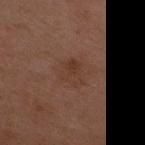Assessment:
Imaged during a routine full-body skin examination; the lesion was not biopsied and no histopathology is available.
Image and clinical context:
Approximately 3 mm at its widest. A 15 mm crop from a total-body photograph taken for skin-cancer surveillance. The patient is a female aged around 35. The tile uses white-light illumination. The lesion is located on the chest. The total-body-photography lesion software estimated a lesion area of about 6 mm², an eccentricity of roughly 0.6, and a symmetry-axis asymmetry near 0.35. The software also gave a mean CIELAB color near L≈37 a*≈19 b*≈27, a lesion–skin lightness drop of about 5, and a lesion-to-skin contrast of about 5.5 (normalized; higher = more distinct).